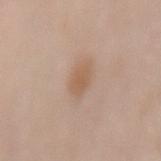<case>
  <biopsy_status>not biopsied; imaged during a skin examination</biopsy_status>
  <site>back</site>
  <lighting>white-light</lighting>
  <patient>
    <sex>female</sex>
    <age_approx>50</age_approx>
  </patient>
  <image>
    <source>total-body photography crop</source>
    <field_of_view_mm>15</field_of_view_mm>
  </image>
</case>